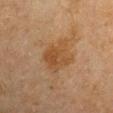Case summary:
- workup · catalogued during a skin exam; not biopsied
- body site · the left upper arm
- image source · ~15 mm tile from a whole-body skin photo
- subject · male, roughly 65 years of age